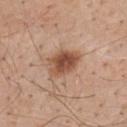| feature | finding |
|---|---|
| notes | imaged on a skin check; not biopsied |
| image source | total-body-photography crop, ~15 mm field of view |
| lighting | white-light |
| subject | male, about 55 years old |
| location | the mid back |